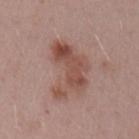A close-up tile cropped from a whole-body skin photograph, about 15 mm across. The recorded lesion diameter is about 6.5 mm. A female patient, in their mid- to late 40s. This is a white-light tile. The lesion is on the left upper arm. The total-body-photography lesion software estimated an average lesion color of about L≈50 a*≈21 b*≈25 (CIELAB), roughly 10 lightness units darker than nearby skin, and a normalized lesion–skin contrast near 7.5.